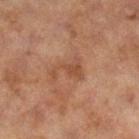{"biopsy_status": "not biopsied; imaged during a skin examination", "image": {"source": "total-body photography crop", "field_of_view_mm": 15}, "site": "right lower leg", "lesion_size": {"long_diameter_mm_approx": 4.0}, "automated_metrics": {"nevus_likeness_0_100": 0}, "lighting": "cross-polarized", "patient": {"sex": "female", "age_approx": 60}}